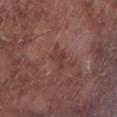Q: Who is the patient?
A: male, approximately 75 years of age
Q: What is the anatomic site?
A: the left lower leg
Q: What is the imaging modality?
A: ~15 mm tile from a whole-body skin photo